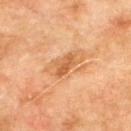Clinical impression:
No biopsy was performed on this lesion — it was imaged during a full skin examination and was not determined to be concerning.
Image and clinical context:
Cropped from a whole-body photographic skin survey; the tile spans about 15 mm. The lesion is on the back. Approximately 3.5 mm at its widest. This is a cross-polarized tile. A male subject aged approximately 75.